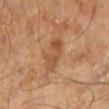Impression: Imaged during a routine full-body skin examination; the lesion was not biopsied and no histopathology is available. Background: The lesion is on the left lower leg. A male patient, in their mid-40s. Imaged with cross-polarized lighting. A roughly 15 mm field-of-view crop from a total-body skin photograph. Approximately 4 mm at its widest.